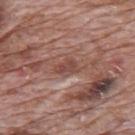No biopsy was performed on this lesion — it was imaged during a full skin examination and was not determined to be concerning.
A male patient, in their 70s.
On the mid back.
Imaged with white-light lighting.
A lesion tile, about 15 mm wide, cut from a 3D total-body photograph.
Approximately 3 mm at its widest.